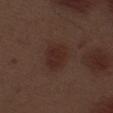Clinical impression:
Recorded during total-body skin imaging; not selected for excision or biopsy.
Image and clinical context:
The lesion is located on the left thigh. This image is a 15 mm lesion crop taken from a total-body photograph. A male subject, about 70 years old.